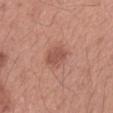The lesion is located on the right forearm. Cropped from a whole-body photographic skin survey; the tile spans about 15 mm. Measured at roughly 3 mm in maximum diameter. A female subject aged around 40.